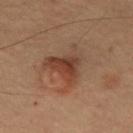Findings:
– follow-up · catalogued during a skin exam; not biopsied
– illumination · cross-polarized illumination
– lesion diameter · ~6.5 mm (longest diameter)
– anatomic site · the chest
– subject · male, about 55 years old
– image source · ~15 mm tile from a whole-body skin photo